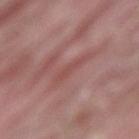subject: male, aged 38–42
automated metrics: a shape eccentricity near 0.9 and two-axis asymmetry of about 0.35; an average lesion color of about L≈49 a*≈25 b*≈23 (CIELAB), about 6 CIELAB-L* units darker than the surrounding skin, and a normalized border contrast of about 5; a nevus-likeness score of about 0/100 and lesion-presence confidence of about 65/100
image: 15 mm crop, total-body photography
body site: the back
illumination: white-light
size: ~3.5 mm (longest diameter)